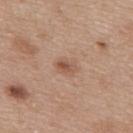notes=imaged on a skin check; not biopsied | site=the back | lesion size=≈2.5 mm | imaging modality=total-body-photography crop, ~15 mm field of view | subject=female, about 40 years old | tile lighting=white-light | automated metrics=a lesion color around L≈54 a*≈20 b*≈29 in CIELAB, about 9 CIELAB-L* units darker than the surrounding skin, and a normalized border contrast of about 6.5; a border-irregularity index near 2.5/10, a color-variation rating of about 4.5/10, and radial color variation of about 1.5.